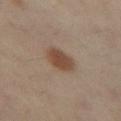Assessment: This lesion was catalogued during total-body skin photography and was not selected for biopsy. Clinical summary: The subject is a female aged 38 to 42. The lesion is on the left thigh. The lesion's longest dimension is about 4 mm. The tile uses cross-polarized illumination. Automated tile analysis of the lesion measured an area of roughly 8 mm² and a symmetry-axis asymmetry near 0.1. The software also gave a border-irregularity rating of about 1.5/10, a within-lesion color-variation index near 3/10, and a peripheral color-asymmetry measure near 1. It also reported a nevus-likeness score of about 100/100 and lesion-presence confidence of about 100/100. A 15 mm crop from a total-body photograph taken for skin-cancer surveillance.